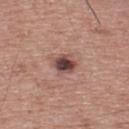{"biopsy_status": "not biopsied; imaged during a skin examination", "site": "upper back", "patient": {"sex": "male", "age_approx": 65}, "lesion_size": {"long_diameter_mm_approx": 3.0}, "image": {"source": "total-body photography crop", "field_of_view_mm": 15}, "lighting": "white-light"}